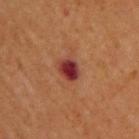This lesion was catalogued during total-body skin photography and was not selected for biopsy.
A close-up tile cropped from a whole-body skin photograph, about 15 mm across.
Approximately 2.5 mm at its widest.
The lesion-visualizer software estimated an area of roughly 5 mm² and an eccentricity of roughly 0.6. The analysis additionally found an average lesion color of about L≈32 a*≈28 b*≈24 (CIELAB), about 13 CIELAB-L* units darker than the surrounding skin, and a lesion-to-skin contrast of about 13 (normalized; higher = more distinct). The software also gave border irregularity of about 2 on a 0–10 scale, a color-variation rating of about 4.5/10, and radial color variation of about 1.5. The software also gave a classifier nevus-likeness of about 0/100 and a detector confidence of about 100 out of 100 that the crop contains a lesion.
A male patient, in their mid- to late 50s.
Captured under cross-polarized illumination.
On the upper back.